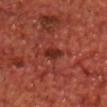<record>
  <site>chest</site>
  <lesion_size>
    <long_diameter_mm_approx>2.5</long_diameter_mm_approx>
  </lesion_size>
  <automated_metrics>
    <area_mm2_approx>3.5</area_mm2_approx>
    <shape_asymmetry>0.25</shape_asymmetry>
  </automated_metrics>
  <image>
    <source>total-body photography crop</source>
    <field_of_view_mm>15</field_of_view_mm>
  </image>
  <lighting>cross-polarized</lighting>
  <patient>
    <sex>male</sex>
    <age_approx>70</age_approx>
  </patient>
</record>